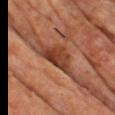Clinical impression:
The lesion was photographed on a routine skin check and not biopsied; there is no pathology result.
Image and clinical context:
A 15 mm crop from a total-body photograph taken for skin-cancer surveillance. A male subject, approximately 60 years of age. The lesion is on the back. The lesion's longest dimension is about 5.5 mm. The tile uses cross-polarized illumination.